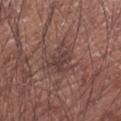<lesion>
<biopsy_status>not biopsied; imaged during a skin examination</biopsy_status>
<patient>
  <sex>male</sex>
  <age_approx>65</age_approx>
</patient>
<site>left upper arm</site>
<lighting>white-light</lighting>
<lesion_size>
  <long_diameter_mm_approx>3.0</long_diameter_mm_approx>
</lesion_size>
<image>
  <source>total-body photography crop</source>
  <field_of_view_mm>15</field_of_view_mm>
</image>
</lesion>